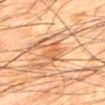Case summary:
• workup · no biopsy performed (imaged during a skin exam)
• patient · male, aged around 65
• image-analysis metrics · a lesion area of about 5 mm², an outline eccentricity of about 0.9 (0 = round, 1 = elongated), and two-axis asymmetry of about 0.4; a mean CIELAB color near L≈49 a*≈22 b*≈35, a lesion–skin lightness drop of about 8, and a normalized border contrast of about 6.5; border irregularity of about 4.5 on a 0–10 scale, internal color variation of about 2.5 on a 0–10 scale, and a peripheral color-asymmetry measure near 0.5; a lesion-detection confidence of about 90/100
• site · the mid back
• acquisition · ~15 mm tile from a whole-body skin photo
• diameter · ≈4 mm
• lighting · cross-polarized illumination